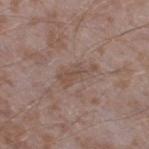biopsy_status: not biopsied; imaged during a skin examination
patient:
  sex: male
  age_approx: 45
lighting: white-light
automated_metrics:
  area_mm2_approx: 6.0
  eccentricity: 0.9
  shape_asymmetry: 0.4
  cielab_L: 49
  cielab_a: 16
  cielab_b: 24
  vs_skin_darker_L: 7.0
  border_irregularity_0_10: 5.0
  peripheral_color_asymmetry: 1.0
  nevus_likeness_0_100: 0
  lesion_detection_confidence_0_100: 95
image:
  source: total-body photography crop
  field_of_view_mm: 15
site: right thigh